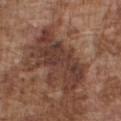workup — total-body-photography surveillance lesion; no biopsy
image — 15 mm crop, total-body photography
site — the chest
patient — male, roughly 75 years of age